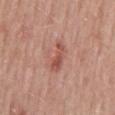Assessment: The lesion was photographed on a routine skin check and not biopsied; there is no pathology result. Context: From the back. Cropped from a total-body skin-imaging series; the visible field is about 15 mm. The subject is a male aged around 50.